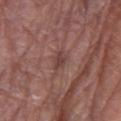follow-up: total-body-photography surveillance lesion; no biopsy
image source: total-body-photography crop, ~15 mm field of view
anatomic site: the left upper arm
patient: male, approximately 80 years of age